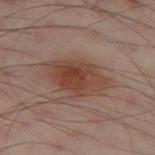Part of a total-body skin-imaging series; this lesion was reviewed on a skin check and was not flagged for biopsy.
The lesion is on the leg.
A region of skin cropped from a whole-body photographic capture, roughly 15 mm wide.
The recorded lesion diameter is about 6.5 mm.
A male patient aged around 50.
Captured under cross-polarized illumination.
Automated tile analysis of the lesion measured a mean CIELAB color near L≈34 a*≈16 b*≈21 and a normalized border contrast of about 8.